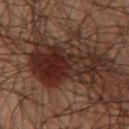| key | value |
|---|---|
| notes | no biopsy performed (imaged during a skin exam) |
| subject | male, aged approximately 60 |
| automated lesion analysis | a lesion area of about 30 mm²; about 8 CIELAB-L* units darker than the surrounding skin and a lesion-to-skin contrast of about 10 (normalized; higher = more distinct) |
| location | the chest |
| image | total-body-photography crop, ~15 mm field of view |
| lighting | cross-polarized illumination |